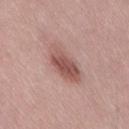The lesion was tiled from a total-body skin photograph and was not biopsied. The lesion is located on the lower back. A roughly 15 mm field-of-view crop from a total-body skin photograph. Longest diameter approximately 5 mm. The subject is a female aged 38–42.